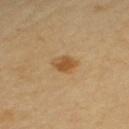Impression:
This lesion was catalogued during total-body skin photography and was not selected for biopsy.
Clinical summary:
The lesion-visualizer software estimated a lesion area of about 4.5 mm², a shape eccentricity near 0.65, and a shape-asymmetry score of about 0.2 (0 = symmetric). The analysis additionally found a mean CIELAB color near L≈53 a*≈18 b*≈40, roughly 10 lightness units darker than nearby skin, and a lesion-to-skin contrast of about 8 (normalized; higher = more distinct). The analysis additionally found border irregularity of about 2 on a 0–10 scale and peripheral color asymmetry of about 1.5. It also reported a nevus-likeness score of about 95/100. The lesion is located on the upper back. Longest diameter approximately 3 mm. A region of skin cropped from a whole-body photographic capture, roughly 15 mm wide. A female patient, aged around 60.